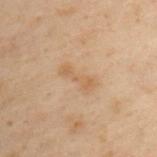No biopsy was performed on this lesion — it was imaged during a full skin examination and was not determined to be concerning. The lesion is located on the upper back. A male subject, aged 48 to 52. This image is a 15 mm lesion crop taken from a total-body photograph. Captured under cross-polarized illumination.